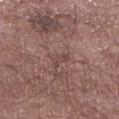Clinical impression: Part of a total-body skin-imaging series; this lesion was reviewed on a skin check and was not flagged for biopsy. Image and clinical context: The lesion's longest dimension is about 3 mm. The subject is a male in their mid-50s. A 15 mm close-up extracted from a 3D total-body photography capture. Located on the right lower leg. An algorithmic analysis of the crop reported a lesion color around L≈45 a*≈19 b*≈21 in CIELAB, a lesion–skin lightness drop of about 6, and a normalized lesion–skin contrast near 5. And it measured an automated nevus-likeness rating near 0 out of 100. Captured under white-light illumination.